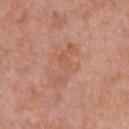Q: Was a biopsy performed?
A: no biopsy performed (imaged during a skin exam)
Q: Lesion location?
A: the chest
Q: How was the tile lit?
A: white-light
Q: How large is the lesion?
A: ~5.5 mm (longest diameter)
Q: What did automated image analysis measure?
A: an average lesion color of about L≈56 a*≈23 b*≈31 (CIELAB), about 6 CIELAB-L* units darker than the surrounding skin, and a normalized border contrast of about 4.5; a border-irregularity index near 8/10, a within-lesion color-variation index near 1.5/10, and a peripheral color-asymmetry measure near 0.5
Q: Patient demographics?
A: male, in their 70s
Q: How was this image acquired?
A: 15 mm crop, total-body photography